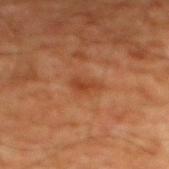<lesion>
<biopsy_status>not biopsied; imaged during a skin examination</biopsy_status>
<lesion_size>
  <long_diameter_mm_approx>3.0</long_diameter_mm_approx>
</lesion_size>
<lighting>cross-polarized</lighting>
<image>
  <source>total-body photography crop</source>
  <field_of_view_mm>15</field_of_view_mm>
</image>
<patient>
  <sex>male</sex>
  <age_approx>60</age_approx>
</patient>
<site>front of the torso</site>
</lesion>